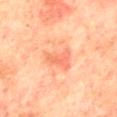<case>
<biopsy_status>not biopsied; imaged during a skin examination</biopsy_status>
<site>mid back</site>
<patient>
  <sex>male</sex>
  <age_approx>75</age_approx>
</patient>
<image>
  <source>total-body photography crop</source>
  <field_of_view_mm>15</field_of_view_mm>
</image>
<automated_metrics>
  <eccentricity>0.7</eccentricity>
  <vs_skin_darker_L>7.0</vs_skin_darker_L>
  <vs_skin_contrast_norm>5.0</vs_skin_contrast_norm>
  <border_irregularity_0_10>4.5</border_irregularity_0_10>
  <color_variation_0_10>4.0</color_variation_0_10>
  <peripheral_color_asymmetry>1.5</peripheral_color_asymmetry>
</automated_metrics>
</case>